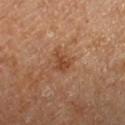Case summary:
– workup: total-body-photography surveillance lesion; no biopsy
– illumination: cross-polarized
– image source: ~15 mm tile from a whole-body skin photo
– image-analysis metrics: a footprint of about 4.5 mm², a shape eccentricity near 0.55, and a symmetry-axis asymmetry near 0.45
– subject: male, roughly 65 years of age
– site: the left forearm